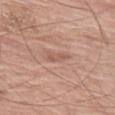biopsy status: imaged on a skin check; not biopsied | patient: female, aged approximately 75 | tile lighting: white-light illumination | image: ~15 mm tile from a whole-body skin photo | automated lesion analysis: an area of roughly 3 mm² and two-axis asymmetry of about 0.25; border irregularity of about 3 on a 0–10 scale, a within-lesion color-variation index near 1.5/10, and peripheral color asymmetry of about 0.5 | location: the left thigh.